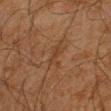This lesion was catalogued during total-body skin photography and was not selected for biopsy. Captured under cross-polarized illumination. The lesion-visualizer software estimated an area of roughly 4 mm², an outline eccentricity of about 0.8 (0 = round, 1 = elongated), and a symmetry-axis asymmetry near 0.3. It also reported a lesion color around L≈32 a*≈17 b*≈27 in CIELAB, about 5 CIELAB-L* units darker than the surrounding skin, and a normalized lesion–skin contrast near 5. It also reported a border-irregularity rating of about 4/10, a color-variation rating of about 1/10, and a peripheral color-asymmetry measure near 0.5. The analysis additionally found an automated nevus-likeness rating near 0 out of 100. A male patient aged around 45. A close-up tile cropped from a whole-body skin photograph, about 15 mm across. The lesion is located on the right upper arm.